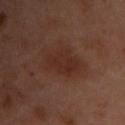The lesion was photographed on a routine skin check and not biopsied; there is no pathology result. Measured at roughly 5.5 mm in maximum diameter. On the chest. Imaged with cross-polarized lighting. A lesion tile, about 15 mm wide, cut from a 3D total-body photograph. A female subject aged approximately 55.